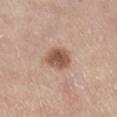Approximately 3.5 mm at its widest. A region of skin cropped from a whole-body photographic capture, roughly 15 mm wide. The lesion is located on the right lower leg. A female patient, aged around 65. Imaged with white-light lighting.